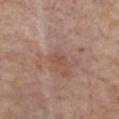{
  "biopsy_status": "not biopsied; imaged during a skin examination",
  "site": "upper back",
  "image": {
    "source": "total-body photography crop",
    "field_of_view_mm": 15
  },
  "lesion_size": {
    "long_diameter_mm_approx": 1.5
  },
  "patient": {
    "sex": "female",
    "age_approx": 75
  },
  "lighting": "cross-polarized"
}